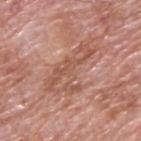– biopsy status — total-body-photography surveillance lesion; no biopsy
– illumination — white-light
– automated metrics — an area of roughly 16 mm², an eccentricity of roughly 0.9, and a symmetry-axis asymmetry near 0.5; an automated nevus-likeness rating near 0 out of 100 and a lesion-detection confidence of about 75/100
– diameter — ~7.5 mm (longest diameter)
– subject — male, roughly 60 years of age
– acquisition — 15 mm crop, total-body photography
– site — the upper back